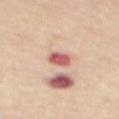Recorded during total-body skin imaging; not selected for excision or biopsy.
The lesion is located on the mid back.
A female patient, roughly 65 years of age.
A region of skin cropped from a whole-body photographic capture, roughly 15 mm wide.
This is a white-light tile.
The lesion's longest dimension is about 3 mm.